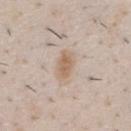Imaged during a routine full-body skin examination; the lesion was not biopsied and no histopathology is available.
The lesion's longest dimension is about 3.5 mm.
The lesion is located on the chest.
A region of skin cropped from a whole-body photographic capture, roughly 15 mm wide.
This is a white-light tile.
A male subject aged around 50.
The lesion-visualizer software estimated a mean CIELAB color near L≈63 a*≈15 b*≈29, roughly 9 lightness units darker than nearby skin, and a lesion-to-skin contrast of about 7 (normalized; higher = more distinct). And it measured a border-irregularity rating of about 2/10, a color-variation rating of about 2.5/10, and a peripheral color-asymmetry measure near 1. And it measured a classifier nevus-likeness of about 95/100 and lesion-presence confidence of about 100/100.